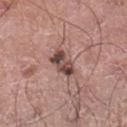Captured during whole-body skin photography for melanoma surveillance; the lesion was not biopsied. Captured under white-light illumination. Cropped from a total-body skin-imaging series; the visible field is about 15 mm. From the left lower leg. A male subject in their 60s.